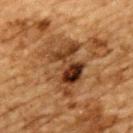Captured during whole-body skin photography for melanoma surveillance; the lesion was not biopsied. The lesion is on the back. Cropped from a whole-body photographic skin survey; the tile spans about 15 mm. A male patient aged approximately 85 or older. Automated image analysis of the tile measured an area of roughly 19 mm², an outline eccentricity of about 0.75 (0 = round, 1 = elongated), and two-axis asymmetry of about 0.4. And it measured about 11 CIELAB-L* units darker than the surrounding skin and a normalized border contrast of about 10. The software also gave border irregularity of about 7.5 on a 0–10 scale, a color-variation rating of about 10/10, and radial color variation of about 4. The software also gave an automated nevus-likeness rating near 0 out of 100 and lesion-presence confidence of about 90/100. This is a cross-polarized tile. Approximately 6.5 mm at its widest.